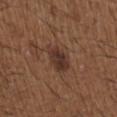Part of a total-body skin-imaging series; this lesion was reviewed on a skin check and was not flagged for biopsy.
A close-up tile cropped from a whole-body skin photograph, about 15 mm across.
The lesion is located on the right upper arm.
A male patient approximately 50 years of age.
Measured at roughly 4 mm in maximum diameter.